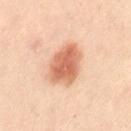Assessment: Captured during whole-body skin photography for melanoma surveillance; the lesion was not biopsied. Background: A 15 mm close-up extracted from a 3D total-body photography capture. From the right leg. A female subject aged approximately 40.